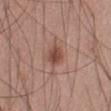Assessment:
The lesion was tiled from a total-body skin photograph and was not biopsied.
Context:
Automated tile analysis of the lesion measured a lesion area of about 5 mm², an outline eccentricity of about 0.5 (0 = round, 1 = elongated), and a symmetry-axis asymmetry near 0.25. And it measured a lesion–skin lightness drop of about 11. Imaged with white-light lighting. Measured at roughly 3 mm in maximum diameter. A male subject, about 55 years old. Located on the abdomen. A region of skin cropped from a whole-body photographic capture, roughly 15 mm wide.